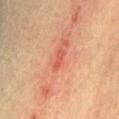{"image": {"source": "total-body photography crop", "field_of_view_mm": 15}, "patient": {"sex": "male", "age_approx": 60}, "site": "abdomen"}